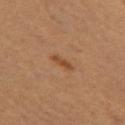Imaged during a routine full-body skin examination; the lesion was not biopsied and no histopathology is available. Located on the left thigh. A roughly 15 mm field-of-view crop from a total-body skin photograph. Captured under cross-polarized illumination. Measured at roughly 2.5 mm in maximum diameter. A female patient in their mid- to late 50s. An algorithmic analysis of the crop reported a mean CIELAB color near L≈48 a*≈23 b*≈36, a lesion–skin lightness drop of about 8, and a lesion-to-skin contrast of about 7 (normalized; higher = more distinct). The software also gave an automated nevus-likeness rating near 50 out of 100 and lesion-presence confidence of about 100/100.